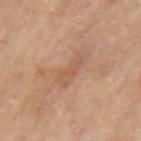No biopsy was performed on this lesion — it was imaged during a full skin examination and was not determined to be concerning.
Imaged with cross-polarized lighting.
The lesion is on the left upper arm.
A lesion tile, about 15 mm wide, cut from a 3D total-body photograph.
The recorded lesion diameter is about 4 mm.
The patient is a female aged approximately 65.